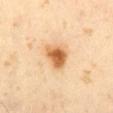Impression: The lesion was tiled from a total-body skin photograph and was not biopsied. Image and clinical context: A male patient, roughly 65 years of age. Captured under cross-polarized illumination. An algorithmic analysis of the crop reported a lesion area of about 8 mm², an outline eccentricity of about 0.7 (0 = round, 1 = elongated), and a shape-asymmetry score of about 0.3 (0 = symmetric). And it measured a lesion color around L≈63 a*≈22 b*≈42 in CIELAB, about 15 CIELAB-L* units darker than the surrounding skin, and a normalized border contrast of about 10. A close-up tile cropped from a whole-body skin photograph, about 15 mm across.